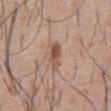Q: Lesion location?
A: the abdomen
Q: What are the patient's age and sex?
A: male, approximately 55 years of age
Q: What is the imaging modality?
A: ~15 mm tile from a whole-body skin photo